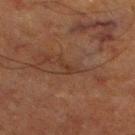Part of a total-body skin-imaging series; this lesion was reviewed on a skin check and was not flagged for biopsy. This is a cross-polarized tile. Cropped from a total-body skin-imaging series; the visible field is about 15 mm. From the right lower leg. A male subject about 65 years old. The lesion-visualizer software estimated a lesion color around L≈28 a*≈16 b*≈24 in CIELAB, a lesion–skin lightness drop of about 5, and a normalized border contrast of about 5. The software also gave a border-irregularity index near 5/10 and radial color variation of about 0. The software also gave a nevus-likeness score of about 0/100 and lesion-presence confidence of about 75/100. The recorded lesion diameter is about 3 mm.